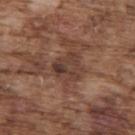| feature | finding |
|---|---|
| lesion size | ~3.5 mm (longest diameter) |
| patient | male, aged 73–77 |
| location | the upper back |
| acquisition | total-body-photography crop, ~15 mm field of view |